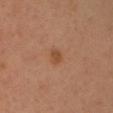No biopsy was performed on this lesion — it was imaged during a full skin examination and was not determined to be concerning. About 2 mm across. The lesion is located on the head or neck. A female patient, approximately 35 years of age. Imaged with cross-polarized lighting. A region of skin cropped from a whole-body photographic capture, roughly 15 mm wide.